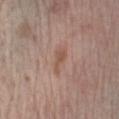biopsy_status: not biopsied; imaged during a skin examination
image:
  source: total-body photography crop
  field_of_view_mm: 15
automated_metrics:
  shape_asymmetry: 0.45
  nevus_likeness_0_100: 0
  lesion_detection_confidence_0_100: 100
site: right forearm
patient:
  sex: female
  age_approx: 70
lighting: white-light